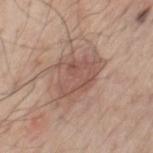workup: no biopsy performed (imaged during a skin exam)
subject: male, about 60 years old
body site: the chest
imaging modality: total-body-photography crop, ~15 mm field of view